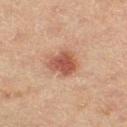  biopsy_status: not biopsied; imaged during a skin examination
  site: right thigh
  lesion_size:
    long_diameter_mm_approx: 3.5
  automated_metrics:
    border_irregularity_0_10: 2.5
    color_variation_0_10: 3.5
  image:
    source: total-body photography crop
    field_of_view_mm: 15
  patient:
    sex: female
    age_approx: 55
  lighting: cross-polarized